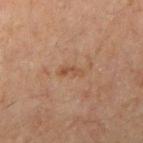biopsy status: no biopsy performed (imaged during a skin exam)
site: the right upper arm
illumination: cross-polarized illumination
TBP lesion metrics: an area of roughly 2 mm², a shape eccentricity near 0.95, and a symmetry-axis asymmetry near 0.45; a classifier nevus-likeness of about 0/100 and a lesion-detection confidence of about 100/100
diameter: about 3 mm
patient: male, aged 43 to 47
image source: 15 mm crop, total-body photography